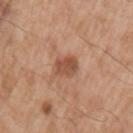tile lighting = white-light; patient = male, aged around 55; acquisition = ~15 mm tile from a whole-body skin photo; location = the left upper arm.